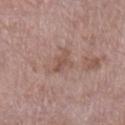The recorded lesion diameter is about 3 mm.
Imaged with white-light lighting.
A female subject aged around 70.
A region of skin cropped from a whole-body photographic capture, roughly 15 mm wide.
From the left lower leg.